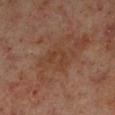Assessment: The lesion was photographed on a routine skin check and not biopsied; there is no pathology result. Background: A 15 mm close-up tile from a total-body photography series done for melanoma screening. From the left lower leg. A male subject aged 58 to 62.